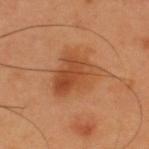Impression:
No biopsy was performed on this lesion — it was imaged during a full skin examination and was not determined to be concerning.
Context:
A close-up tile cropped from a whole-body skin photograph, about 15 mm across. The lesion is on the upper back. Captured under cross-polarized illumination. A male patient about 55 years old. Measured at roughly 6 mm in maximum diameter.